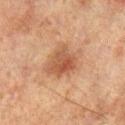Notes:
* notes — total-body-photography surveillance lesion; no biopsy
* automated metrics — a lesion area of about 10 mm² and an eccentricity of roughly 0.6; an average lesion color of about L≈44 a*≈20 b*≈29 (CIELAB), a lesion–skin lightness drop of about 9, and a normalized border contrast of about 7.5; a color-variation rating of about 5.5/10
* site — the leg
* acquisition — 15 mm crop, total-body photography
* patient — male, aged 68–72